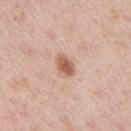Q: Is there a histopathology result?
A: no biopsy performed (imaged during a skin exam)
Q: What are the patient's age and sex?
A: male, aged approximately 40
Q: What lighting was used for the tile?
A: white-light illumination
Q: What did automated image analysis measure?
A: a lesion color around L≈60 a*≈21 b*≈30 in CIELAB and a lesion-to-skin contrast of about 8.5 (normalized; higher = more distinct); an automated nevus-likeness rating near 95 out of 100 and a detector confidence of about 100 out of 100 that the crop contains a lesion
Q: What kind of image is this?
A: total-body-photography crop, ~15 mm field of view
Q: What is the lesion's diameter?
A: ≈3 mm
Q: Lesion location?
A: the right upper arm